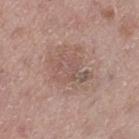Findings:
• workup · total-body-photography surveillance lesion; no biopsy
• image · ~15 mm tile from a whole-body skin photo
• patient · male, in their mid- to late 70s
• lighting · white-light illumination
• automated metrics · an eccentricity of roughly 0.65 and a symmetry-axis asymmetry near 0.3; a mean CIELAB color near L≈54 a*≈17 b*≈23, roughly 7 lightness units darker than nearby skin, and a lesion-to-skin contrast of about 5 (normalized; higher = more distinct); internal color variation of about 3 on a 0–10 scale and a peripheral color-asymmetry measure near 1
• anatomic site · the left thigh
• size · about 5 mm